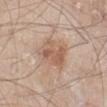Assessment:
The lesion was photographed on a routine skin check and not biopsied; there is no pathology result.
Acquisition and patient details:
Cropped from a whole-body photographic skin survey; the tile spans about 15 mm. A male subject, aged 58 to 62. Captured under white-light illumination. Approximately 4 mm at its widest. The lesion is on the left lower leg. The total-body-photography lesion software estimated a footprint of about 10 mm², an outline eccentricity of about 0.7 (0 = round, 1 = elongated), and a shape-asymmetry score of about 0.35 (0 = symmetric). The software also gave an average lesion color of about L≈58 a*≈19 b*≈29 (CIELAB), about 9 CIELAB-L* units darker than the surrounding skin, and a normalized lesion–skin contrast near 6.5.